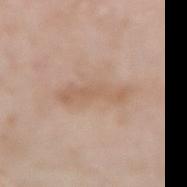Context: A female patient about 70 years old. A 15 mm crop from a total-body photograph taken for skin-cancer surveillance. An algorithmic analysis of the crop reported a lesion area of about 11 mm² and a symmetry-axis asymmetry near 0.3. The analysis additionally found about 7 CIELAB-L* units darker than the surrounding skin and a lesion-to-skin contrast of about 5.5 (normalized; higher = more distinct). From the left forearm. Measured at roughly 6.5 mm in maximum diameter.